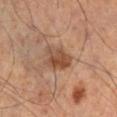biopsy_status: not biopsied; imaged during a skin examination
image:
  source: total-body photography crop
  field_of_view_mm: 15
patient:
  sex: male
  age_approx: 55
site: right lower leg
lesion_size:
  long_diameter_mm_approx: 4.0
automated_metrics:
  area_mm2_approx: 7.5
  eccentricity: 0.8
  shape_asymmetry: 0.2
  border_irregularity_0_10: 3.0
  color_variation_0_10: 4.0
lighting: cross-polarized